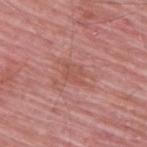notes: total-body-photography surveillance lesion; no biopsy.